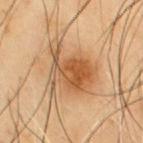image = total-body-photography crop, ~15 mm field of view; location = the chest; lighting = cross-polarized illumination; patient = male, aged 53 to 57; lesion size = ≈6.5 mm.